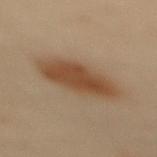<record>
  <biopsy_status>not biopsied; imaged during a skin examination</biopsy_status>
  <patient>
    <sex>female</sex>
    <age_approx>60</age_approx>
  </patient>
  <lighting>cross-polarized</lighting>
  <site>upper back</site>
  <image>
    <source>total-body photography crop</source>
    <field_of_view_mm>15</field_of_view_mm>
  </image>
  <lesion_size>
    <long_diameter_mm_approx>6.5</long_diameter_mm_approx>
  </lesion_size>
</record>